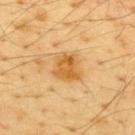Captured during whole-body skin photography for melanoma surveillance; the lesion was not biopsied. About 3.5 mm across. Automated tile analysis of the lesion measured an eccentricity of roughly 0.5 and a symmetry-axis asymmetry near 0.35. The analysis additionally found a lesion color around L≈63 a*≈22 b*≈50 in CIELAB, about 11 CIELAB-L* units darker than the surrounding skin, and a lesion-to-skin contrast of about 8 (normalized; higher = more distinct). A 15 mm crop from a total-body photograph taken for skin-cancer surveillance. This is a cross-polarized tile. The lesion is located on the upper back. The subject is a male roughly 65 years of age.